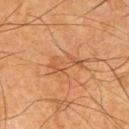Captured during whole-body skin photography for melanoma surveillance; the lesion was not biopsied.
A 15 mm crop from a total-body photograph taken for skin-cancer surveillance.
On the chest.
A male patient in their mid-60s.
An algorithmic analysis of the crop reported border irregularity of about 6.5 on a 0–10 scale and a peripheral color-asymmetry measure near 0.5.
The lesion's longest dimension is about 4.5 mm.
The tile uses cross-polarized illumination.